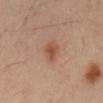| feature | finding |
|---|---|
| follow-up | imaged on a skin check; not biopsied |
| subject | male, aged around 55 |
| acquisition | total-body-photography crop, ~15 mm field of view |
| image-analysis metrics | an outline eccentricity of about 0.8 (0 = round, 1 = elongated); an average lesion color of about L≈49 a*≈21 b*≈30 (CIELAB), a lesion–skin lightness drop of about 8, and a normalized border contrast of about 7; a color-variation rating of about 2.5/10 and radial color variation of about 0.5 |
| site | the abdomen |